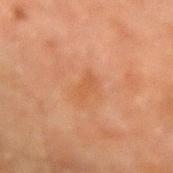Assessment:
Captured during whole-body skin photography for melanoma surveillance; the lesion was not biopsied.
Image and clinical context:
This is a cross-polarized tile. The total-body-photography lesion software estimated an area of roughly 3 mm² and a shape eccentricity near 0.85. The analysis additionally found a border-irregularity rating of about 3.5/10, a color-variation rating of about 0.5/10, and radial color variation of about 0. And it measured a classifier nevus-likeness of about 0/100 and lesion-presence confidence of about 100/100. On the left forearm. Measured at roughly 2.5 mm in maximum diameter. Cropped from a whole-body photographic skin survey; the tile spans about 15 mm. The subject is a male aged approximately 60.